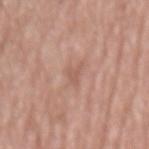{
  "biopsy_status": "not biopsied; imaged during a skin examination",
  "patient": {
    "sex": "female",
    "age_approx": 75
  },
  "lesion_size": {
    "long_diameter_mm_approx": 2.5
  },
  "lighting": "white-light",
  "image": {
    "source": "total-body photography crop",
    "field_of_view_mm": 15
  },
  "site": "mid back"
}